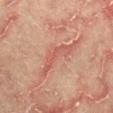biopsy status: total-body-photography surveillance lesion; no biopsy | image-analysis metrics: a lesion area of about 9 mm² and two-axis asymmetry of about 0.7; a color-variation rating of about 2.5/10 and peripheral color asymmetry of about 1 | location: the front of the torso | subject: male, aged 63 to 67 | lesion diameter: about 6.5 mm | image: total-body-photography crop, ~15 mm field of view | illumination: cross-polarized illumination.